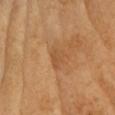{"biopsy_status": "not biopsied; imaged during a skin examination", "patient": {"sex": "female", "age_approx": 65}, "site": "head or neck", "automated_metrics": {"area_mm2_approx": 3.5, "eccentricity": 0.65, "shape_asymmetry": 0.4, "cielab_L": 51, "cielab_a": 22, "cielab_b": 38, "nevus_likeness_0_100": 0, "lesion_detection_confidence_0_100": 100}, "lighting": "cross-polarized", "image": {"source": "total-body photography crop", "field_of_view_mm": 15}, "lesion_size": {"long_diameter_mm_approx": 2.5}}